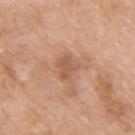{"biopsy_status": "not biopsied; imaged during a skin examination", "lighting": "white-light", "patient": {"sex": "female", "age_approx": 75}, "lesion_size": {"long_diameter_mm_approx": 2.5}, "site": "right upper arm", "image": {"source": "total-body photography crop", "field_of_view_mm": 15}}